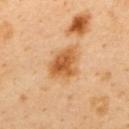Part of a total-body skin-imaging series; this lesion was reviewed on a skin check and was not flagged for biopsy.
The lesion-visualizer software estimated a lesion area of about 12 mm² and a symmetry-axis asymmetry near 0.3. And it measured a lesion color around L≈60 a*≈24 b*≈43 in CIELAB, about 12 CIELAB-L* units darker than the surrounding skin, and a normalized border contrast of about 8.5. And it measured a border-irregularity index near 2.5/10.
A male patient about 40 years old.
A 15 mm close-up tile from a total-body photography series done for melanoma screening.
From the back.
Imaged with cross-polarized lighting.